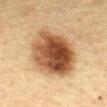Clinical summary:
Captured under cross-polarized illumination. The lesion is located on the chest. A close-up tile cropped from a whole-body skin photograph, about 15 mm across. A female patient, aged approximately 55.